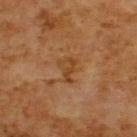{
  "lighting": "cross-polarized",
  "patient": {
    "sex": "male",
    "age_approx": 65
  },
  "lesion_size": {
    "long_diameter_mm_approx": 3.0
  },
  "image": {
    "source": "total-body photography crop",
    "field_of_view_mm": 15
  },
  "automated_metrics": {
    "cielab_L": 42,
    "cielab_a": 22,
    "cielab_b": 37,
    "vs_skin_darker_L": 7.0,
    "vs_skin_contrast_norm": 6.5
  },
  "site": "upper back"
}